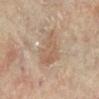Clinical impression: This lesion was catalogued during total-body skin photography and was not selected for biopsy. Background: The patient is a female aged approximately 80. The lesion is located on the right lower leg. Captured under cross-polarized illumination. Automated image analysis of the tile measured a lesion area of about 11 mm², a shape eccentricity near 0.65, and a shape-asymmetry score of about 0.4 (0 = symmetric). It also reported a lesion–skin lightness drop of about 6 and a lesion-to-skin contrast of about 5 (normalized; higher = more distinct). A region of skin cropped from a whole-body photographic capture, roughly 15 mm wide. The lesion's longest dimension is about 4.5 mm.